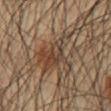Clinical impression:
No biopsy was performed on this lesion — it was imaged during a full skin examination and was not determined to be concerning.
Image and clinical context:
A 15 mm close-up extracted from a 3D total-body photography capture. Automated tile analysis of the lesion measured a lesion area of about 15 mm² and a symmetry-axis asymmetry near 0.5. About 6.5 mm across. On the abdomen. A male subject aged around 60.